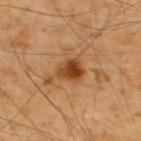Assessment:
Recorded during total-body skin imaging; not selected for excision or biopsy.
Clinical summary:
A 15 mm crop from a total-body photograph taken for skin-cancer surveillance. Imaged with cross-polarized lighting. The patient is a male in their mid-50s. The recorded lesion diameter is about 3 mm. The lesion is located on the back. Automated image analysis of the tile measured a footprint of about 6 mm², an outline eccentricity of about 0.6 (0 = round, 1 = elongated), and a symmetry-axis asymmetry near 0.25. The software also gave an average lesion color of about L≈42 a*≈24 b*≈38 (CIELAB), about 13 CIELAB-L* units darker than the surrounding skin, and a normalized lesion–skin contrast near 10. And it measured border irregularity of about 2.5 on a 0–10 scale and radial color variation of about 2. The analysis additionally found an automated nevus-likeness rating near 95 out of 100.